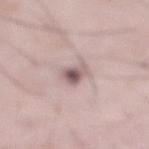Notes:
- biopsy status · no biopsy performed (imaged during a skin exam)
- subject · male, in their mid- to late 50s
- body site · the abdomen
- imaging modality · total-body-photography crop, ~15 mm field of view